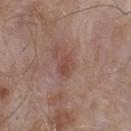Impression: Imaged during a routine full-body skin examination; the lesion was not biopsied and no histopathology is available. Background: Cropped from a whole-body photographic skin survey; the tile spans about 15 mm. On the upper back. About 2.5 mm across. The tile uses white-light illumination. An algorithmic analysis of the crop reported a lesion area of about 3.5 mm², a shape eccentricity near 0.75, and two-axis asymmetry of about 0.25. The software also gave a lesion color around L≈47 a*≈21 b*≈24 in CIELAB. It also reported a border-irregularity index near 2.5/10 and a peripheral color-asymmetry measure near 1. The software also gave an automated nevus-likeness rating near 0 out of 100 and a detector confidence of about 100 out of 100 that the crop contains a lesion. The patient is a male in their mid- to late 50s.